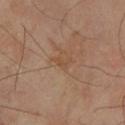Part of a total-body skin-imaging series; this lesion was reviewed on a skin check and was not flagged for biopsy.
Approximately 2.5 mm at its widest.
Captured under cross-polarized illumination.
A male subject, in their mid- to late 60s.
On the left lower leg.
Cropped from a whole-body photographic skin survey; the tile spans about 15 mm.